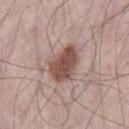workup = imaged on a skin check; not biopsied